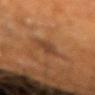The lesion was photographed on a routine skin check and not biopsied; there is no pathology result.
The lesion-visualizer software estimated border irregularity of about 4 on a 0–10 scale, internal color variation of about 1 on a 0–10 scale, and a peripheral color-asymmetry measure near 0.5.
Located on the left forearm.
A male subject aged around 60.
A roughly 15 mm field-of-view crop from a total-body skin photograph.